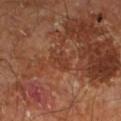Q: Is there a histopathology result?
A: no biopsy performed (imaged during a skin exam)
Q: What are the patient's age and sex?
A: male, aged 58 to 62
Q: Lesion location?
A: the leg
Q: How was this image acquired?
A: ~15 mm tile from a whole-body skin photo
Q: Automated lesion metrics?
A: an area of roughly 3.5 mm²; an average lesion color of about L≈36 a*≈23 b*≈30 (CIELAB), a lesion–skin lightness drop of about 6, and a normalized lesion–skin contrast near 5; a border-irregularity rating of about 3.5/10, a within-lesion color-variation index near 1/10, and a peripheral color-asymmetry measure near 0
Q: Lesion size?
A: about 3 mm